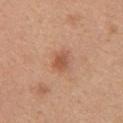  biopsy_status: not biopsied; imaged during a skin examination
  automated_metrics:
    border_irregularity_0_10: 2.5
    color_variation_0_10: 1.5
    peripheral_color_asymmetry: 0.5
    nevus_likeness_0_100: 65
    lesion_detection_confidence_0_100: 100
  patient:
    sex: female
    age_approx: 45
  site: upper back
  image:
    source: total-body photography crop
    field_of_view_mm: 15
  lighting: white-light
  lesion_size:
    long_diameter_mm_approx: 2.5